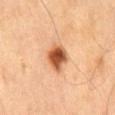Part of a total-body skin-imaging series; this lesion was reviewed on a skin check and was not flagged for biopsy. A close-up tile cropped from a whole-body skin photograph, about 15 mm across. The lesion is on the mid back. Imaged with cross-polarized lighting. Automated tile analysis of the lesion measured a footprint of about 7 mm², a shape eccentricity near 0.55, and a shape-asymmetry score of about 0.2 (0 = symmetric). And it measured a lesion–skin lightness drop of about 19 and a normalized border contrast of about 12. It also reported radial color variation of about 1.5. And it measured a classifier nevus-likeness of about 100/100 and a lesion-detection confidence of about 100/100. Measured at roughly 3.5 mm in maximum diameter. A male patient, approximately 60 years of age.